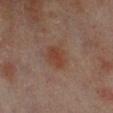Captured during whole-body skin photography for melanoma surveillance; the lesion was not biopsied. The lesion is located on the left lower leg. A female subject roughly 80 years of age. A lesion tile, about 15 mm wide, cut from a 3D total-body photograph.